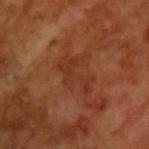Notes:
• workup — catalogued during a skin exam; not biopsied
• diameter — ~4.5 mm (longest diameter)
• image-analysis metrics — a footprint of about 8 mm², an eccentricity of roughly 0.8, and a symmetry-axis asymmetry near 0.5; border irregularity of about 8.5 on a 0–10 scale, internal color variation of about 1 on a 0–10 scale, and radial color variation of about 0.5
• patient — male, in their mid- to late 60s
• lighting — cross-polarized
• acquisition — 15 mm crop, total-body photography